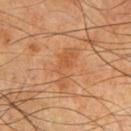Q: Was a biopsy performed?
A: total-body-photography surveillance lesion; no biopsy
Q: Lesion location?
A: the chest
Q: What is the imaging modality?
A: ~15 mm tile from a whole-body skin photo
Q: Illumination type?
A: cross-polarized illumination
Q: How large is the lesion?
A: ≈5 mm
Q: Patient demographics?
A: male, in their mid- to late 60s